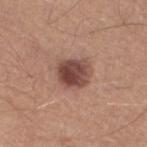workup=no biopsy performed (imaged during a skin exam); location=the leg; lighting=white-light illumination; image=15 mm crop, total-body photography; lesion diameter=~4 mm (longest diameter); patient=male, aged 53–57; TBP lesion metrics=an average lesion color of about L≈46 a*≈21 b*≈24 (CIELAB) and about 14 CIELAB-L* units darker than the surrounding skin.